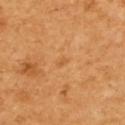Assessment:
The lesion was photographed on a routine skin check and not biopsied; there is no pathology result.
Image and clinical context:
From the upper back. Longest diameter approximately 1 mm. The subject is a female in their mid-50s. A 15 mm close-up tile from a total-body photography series done for melanoma screening.